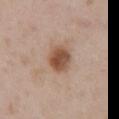<lesion>
  <automated_metrics>
    <border_irregularity_0_10>2.0</border_irregularity_0_10>
    <color_variation_0_10>4.0</color_variation_0_10>
    <peripheral_color_asymmetry>1.5</peripheral_color_asymmetry>
    <nevus_likeness_0_100>100</nevus_likeness_0_100>
    <lesion_detection_confidence_0_100>100</lesion_detection_confidence_0_100>
  </automated_metrics>
  <patient>
    <sex>male</sex>
    <age_approx>55</age_approx>
  </patient>
  <lighting>white-light</lighting>
  <site>chest</site>
  <image>
    <source>total-body photography crop</source>
    <field_of_view_mm>15</field_of_view_mm>
  </image>
</lesion>